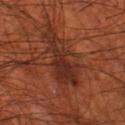Notes:
* anatomic site — the left thigh
* patient — male, about 70 years old
* acquisition — ~15 mm tile from a whole-body skin photo
* lighting — cross-polarized illumination
* lesion diameter — ≈7 mm
* image-analysis metrics — a footprint of about 12 mm², a shape eccentricity near 0.95, and a shape-asymmetry score of about 0.65 (0 = symmetric); peripheral color asymmetry of about 1; an automated nevus-likeness rating near 0 out of 100 and a detector confidence of about 100 out of 100 that the crop contains a lesion